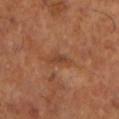biopsy status: no biopsy performed (imaged during a skin exam)
anatomic site: the left lower leg
lesion diameter: ≈3 mm
patient: male, aged around 65
image source: total-body-photography crop, ~15 mm field of view
lighting: cross-polarized illumination